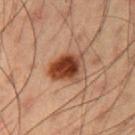notes = imaged on a skin check; not biopsied
anatomic site = the arm
subject = male, approximately 55 years of age
image source = 15 mm crop, total-body photography